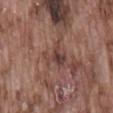follow-up: catalogued during a skin exam; not biopsied.